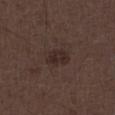  biopsy_status: not biopsied; imaged during a skin examination
  patient:
    sex: male
    age_approx: 50
  image:
    source: total-body photography crop
    field_of_view_mm: 15
  lesion_size:
    long_diameter_mm_approx: 3.0
  automated_metrics:
    area_mm2_approx: 5.5
    eccentricity: 0.7
    shape_asymmetry: 0.25
    cielab_L: 26
    cielab_a: 14
    cielab_b: 17
    vs_skin_darker_L: 6.0
    vs_skin_contrast_norm: 7.0
    border_irregularity_0_10: 3.0
    color_variation_0_10: 2.5
    peripheral_color_asymmetry: 1.0
    nevus_likeness_0_100: 55
    lesion_detection_confidence_0_100: 100
  lighting: white-light
  site: leg